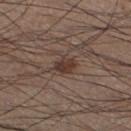biopsy status: no biopsy performed (imaged during a skin exam); patient: male, aged around 35; automated lesion analysis: a footprint of about 4.5 mm², an eccentricity of roughly 0.65, and a symmetry-axis asymmetry near 0.25; illumination: white-light; image source: ~15 mm crop, total-body skin-cancer survey; diameter: ≈2.5 mm; anatomic site: the left lower leg.